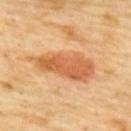{"image": {"source": "total-body photography crop", "field_of_view_mm": 15}, "patient": {"sex": "female", "age_approx": 60}, "site": "upper back", "automated_metrics": {"cielab_L": 63, "cielab_a": 26, "cielab_b": 43, "vs_skin_darker_L": 12.0, "vs_skin_contrast_norm": 7.5}}